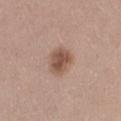follow-up=total-body-photography surveillance lesion; no biopsy | imaging modality=~15 mm tile from a whole-body skin photo | patient=female, aged 23 to 27 | size=about 3 mm | body site=the left thigh.